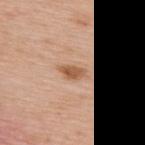patient = female, roughly 40 years of age | image source = ~15 mm tile from a whole-body skin photo | site = the upper back | size = ~3 mm (longest diameter) | tile lighting = white-light illumination.